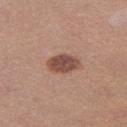workup: no biopsy performed (imaged during a skin exam); image: total-body-photography crop, ~15 mm field of view; illumination: white-light; location: the leg; patient: female, aged around 35.